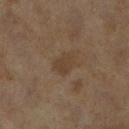Findings:
* site: the leg
* subject: female, aged approximately 60
* lighting: cross-polarized
* imaging modality: ~15 mm tile from a whole-body skin photo
* lesion diameter: ~2.5 mm (longest diameter)